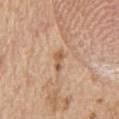Assessment:
Recorded during total-body skin imaging; not selected for excision or biopsy.
Context:
A roughly 15 mm field-of-view crop from a total-body skin photograph. Automated image analysis of the tile measured an average lesion color of about L≈59 a*≈19 b*≈34 (CIELAB), a lesion–skin lightness drop of about 9, and a normalized border contrast of about 6.5. It also reported border irregularity of about 3.5 on a 0–10 scale, internal color variation of about 1.5 on a 0–10 scale, and radial color variation of about 0.5. The recorded lesion diameter is about 2.5 mm. A male patient about 70 years old. Located on the abdomen.